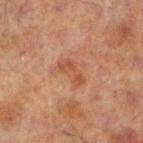Clinical impression: The lesion was photographed on a routine skin check and not biopsied; there is no pathology result. Context: This image is a 15 mm lesion crop taken from a total-body photograph. The lesion is located on the left lower leg. Captured under cross-polarized illumination. Longest diameter approximately 3.5 mm. The lesion-visualizer software estimated an area of roughly 4.5 mm², an eccentricity of roughly 0.9, and a shape-asymmetry score of about 0.45 (0 = symmetric). And it measured an automated nevus-likeness rating near 0 out of 100 and a detector confidence of about 100 out of 100 that the crop contains a lesion. The subject is a male approximately 70 years of age.